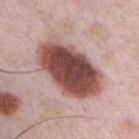notes — imaged on a skin check; not biopsied
image — ~15 mm crop, total-body skin-cancer survey
patient — male, in their mid- to late 30s
illumination — white-light illumination
TBP lesion metrics — an average lesion color of about L≈48 a*≈23 b*≈23 (CIELAB), a lesion–skin lightness drop of about 21, and a normalized border contrast of about 13.5; a classifier nevus-likeness of about 95/100
diameter — ≈8.5 mm
location — the chest